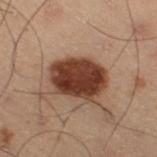follow-up: no biopsy performed (imaged during a skin exam) | acquisition: 15 mm crop, total-body photography | site: the right thigh | lighting: cross-polarized illumination | subject: male, aged 53–57 | lesion size: ≈6 mm.